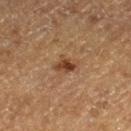Impression: Part of a total-body skin-imaging series; this lesion was reviewed on a skin check and was not flagged for biopsy. Background: The lesion is located on the leg. A region of skin cropped from a whole-body photographic capture, roughly 15 mm wide. The patient is a male approximately 75 years of age.